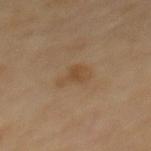Image and clinical context:
The subject is a male aged 68 to 72. Automated tile analysis of the lesion measured a mean CIELAB color near L≈46 a*≈15 b*≈32, roughly 6 lightness units darker than nearby skin, and a lesion-to-skin contrast of about 5.5 (normalized; higher = more distinct). The software also gave internal color variation of about 2 on a 0–10 scale and peripheral color asymmetry of about 0.5. The lesion's longest dimension is about 3 mm. This is a cross-polarized tile. Located on the mid back. A 15 mm close-up tile from a total-body photography series done for melanoma screening.